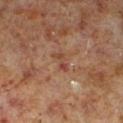Findings:
* biopsy status — imaged on a skin check; not biopsied
* patient — male, aged 58–62
* size — about 2.5 mm
* image source — ~15 mm tile from a whole-body skin photo
* anatomic site — the left lower leg